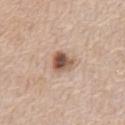Recorded during total-body skin imaging; not selected for excision or biopsy.
The patient is a female aged 58–62.
Located on the chest.
The lesion-visualizer software estimated a shape eccentricity near 0.35 and two-axis asymmetry of about 0.3. The analysis additionally found border irregularity of about 2.5 on a 0–10 scale, a color-variation rating of about 8.5/10, and peripheral color asymmetry of about 2.5.
A close-up tile cropped from a whole-body skin photograph, about 15 mm across.
Imaged with white-light lighting.
Measured at roughly 2.5 mm in maximum diameter.